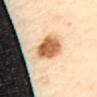Q: Was this lesion biopsied?
A: catalogued during a skin exam; not biopsied
Q: What are the patient's age and sex?
A: female, roughly 65 years of age
Q: Lesion location?
A: the mid back
Q: Automated lesion metrics?
A: a lesion area of about 11 mm², an eccentricity of roughly 0.8, and a symmetry-axis asymmetry near 0.2; a mean CIELAB color near L≈65 a*≈23 b*≈41 and a lesion-to-skin contrast of about 10.5 (normalized; higher = more distinct); a nevus-likeness score of about 100/100 and a lesion-detection confidence of about 100/100
Q: What is the imaging modality?
A: ~15 mm crop, total-body skin-cancer survey